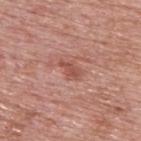The lesion was tiled from a total-body skin photograph and was not biopsied. A male subject in their mid- to late 70s. Approximately 3 mm at its widest. An algorithmic analysis of the crop reported an eccentricity of roughly 0.85. The software also gave an automated nevus-likeness rating near 0 out of 100. A region of skin cropped from a whole-body photographic capture, roughly 15 mm wide. Located on the upper back.